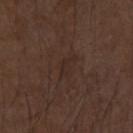Case summary:
- lesion size · ≈3 mm
- image source · ~15 mm crop, total-body skin-cancer survey
- subject · male, approximately 75 years of age
- anatomic site · the right forearm